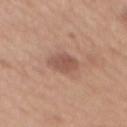{"biopsy_status": "not biopsied; imaged during a skin examination", "image": {"source": "total-body photography crop", "field_of_view_mm": 15}, "site": "mid back", "lesion_size": {"long_diameter_mm_approx": 3.0}, "patient": {"sex": "female", "age_approx": 40}, "lighting": "white-light"}